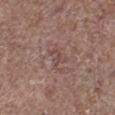Q: Is there a histopathology result?
A: imaged on a skin check; not biopsied
Q: Who is the patient?
A: male, aged 68 to 72
Q: Where on the body is the lesion?
A: the right lower leg
Q: How was this image acquired?
A: total-body-photography crop, ~15 mm field of view